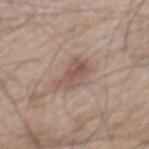notes: catalogued during a skin exam; not biopsied
acquisition: ~15 mm crop, total-body skin-cancer survey
tile lighting: white-light
site: the mid back
diameter: ~4 mm (longest diameter)
patient: male, in their mid- to late 70s